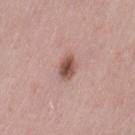Part of a total-body skin-imaging series; this lesion was reviewed on a skin check and was not flagged for biopsy.
The lesion-visualizer software estimated an area of roughly 5 mm². The analysis additionally found a border-irregularity rating of about 1.5/10, internal color variation of about 4 on a 0–10 scale, and a peripheral color-asymmetry measure near 1.5. The analysis additionally found a nevus-likeness score of about 95/100 and a lesion-detection confidence of about 100/100.
From the lower back.
A close-up tile cropped from a whole-body skin photograph, about 15 mm across.
The subject is a female aged 38–42.
Captured under white-light illumination.
Longest diameter approximately 3 mm.